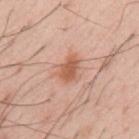Impression:
No biopsy was performed on this lesion — it was imaged during a full skin examination and was not determined to be concerning.
Acquisition and patient details:
Located on the mid back. The total-body-photography lesion software estimated a border-irregularity rating of about 3/10, a within-lesion color-variation index near 2.5/10, and peripheral color asymmetry of about 1. The software also gave lesion-presence confidence of about 100/100. A region of skin cropped from a whole-body photographic capture, roughly 15 mm wide. A male patient, roughly 55 years of age. The tile uses white-light illumination.